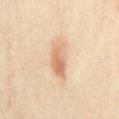- size: ~5.5 mm (longest diameter)
- anatomic site: the mid back
- acquisition: ~15 mm tile from a whole-body skin photo
- subject: female, roughly 35 years of age